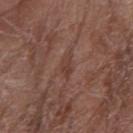Impression: The lesion was tiled from a total-body skin photograph and was not biopsied. Background: A female patient roughly 80 years of age. Located on the right forearm. A 15 mm crop from a total-body photograph taken for skin-cancer surveillance.